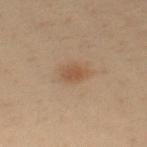Part of a total-body skin-imaging series; this lesion was reviewed on a skin check and was not flagged for biopsy. A male patient in their 50s. This image is a 15 mm lesion crop taken from a total-body photograph. Located on the upper back.